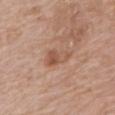Image and clinical context: A 15 mm crop from a total-body photograph taken for skin-cancer surveillance. The tile uses white-light illumination. The patient is a female aged approximately 75. Longest diameter approximately 4 mm. From the mid back.